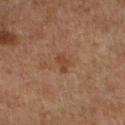{
  "biopsy_status": "not biopsied; imaged during a skin examination",
  "lighting": "cross-polarized",
  "site": "right thigh",
  "patient": {
    "sex": "female",
    "age_approx": 60
  },
  "image": {
    "source": "total-body photography crop",
    "field_of_view_mm": 15
  },
  "lesion_size": {
    "long_diameter_mm_approx": 2.5
  }
}